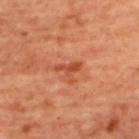{"biopsy_status": "not biopsied; imaged during a skin examination", "site": "upper back", "image": {"source": "total-body photography crop", "field_of_view_mm": 15}, "patient": {"sex": "female", "age_approx": 45}}